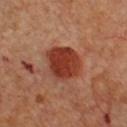Impression:
Imaged during a routine full-body skin examination; the lesion was not biopsied and no histopathology is available.
Image and clinical context:
From the chest. A 15 mm close-up tile from a total-body photography series done for melanoma screening. Imaged with cross-polarized lighting. A male patient, approximately 50 years of age. Automated tile analysis of the lesion measured an area of roughly 15 mm², an outline eccentricity of about 0.55 (0 = round, 1 = elongated), and a symmetry-axis asymmetry near 0.15. It also reported a lesion color around L≈38 a*≈30 b*≈32 in CIELAB, roughly 13 lightness units darker than nearby skin, and a lesion-to-skin contrast of about 10.5 (normalized; higher = more distinct). The software also gave lesion-presence confidence of about 100/100.